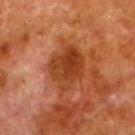follow-up: no biopsy performed (imaged during a skin exam); lighting: cross-polarized illumination; subject: male, about 80 years old; imaging modality: 15 mm crop, total-body photography; size: ~5 mm (longest diameter); location: the left lower leg.